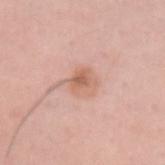  automated_metrics:
    cielab_L: 63
    cielab_a: 22
    cielab_b: 30
    vs_skin_darker_L: 9.0
    vs_skin_contrast_norm: 6.5
    nevus_likeness_0_100: 25
    lesion_detection_confidence_0_100: 100
  lighting: white-light
  site: left forearm
  patient:
    sex: female
    age_approx: 55
  lesion_size:
    long_diameter_mm_approx: 3.5
  image:
    source: total-body photography crop
    field_of_view_mm: 15